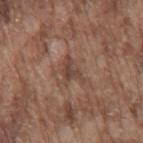Findings:
- image-analysis metrics · an average lesion color of about L≈43 a*≈18 b*≈24 (CIELAB), roughly 8 lightness units darker than nearby skin, and a lesion-to-skin contrast of about 7 (normalized; higher = more distinct)
- image · 15 mm crop, total-body photography
- patient · male, approximately 75 years of age
- body site · the mid back